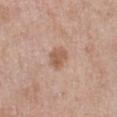<lesion>
  <biopsy_status>not biopsied; imaged during a skin examination</biopsy_status>
  <lighting>white-light</lighting>
  <patient>
    <sex>female</sex>
    <age_approx>40</age_approx>
  </patient>
  <image>
    <source>total-body photography crop</source>
    <field_of_view_mm>15</field_of_view_mm>
  </image>
  <site>left lower leg</site>
  <automated_metrics>
    <area_mm2_approx>5.0</area_mm2_approx>
    <eccentricity>0.65</eccentricity>
    <shape_asymmetry>0.2</shape_asymmetry>
    <cielab_L>57</cielab_L>
    <cielab_a>20</cielab_a>
    <cielab_b>30</cielab_b>
    <vs_skin_darker_L>10.0</vs_skin_darker_L>
    <vs_skin_contrast_norm>7.5</vs_skin_contrast_norm>
    <border_irregularity_0_10>1.5</border_irregularity_0_10>
    <nevus_likeness_0_100>40</nevus_likeness_0_100>
    <lesion_detection_confidence_0_100>100</lesion_detection_confidence_0_100>
  </automated_metrics>
</lesion>